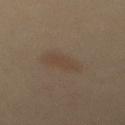biopsy status = total-body-photography surveillance lesion; no biopsy | automated metrics = a lesion area of about 6.5 mm² and a shape eccentricity near 0.9; a lesion–skin lightness drop of about 5 and a normalized border contrast of about 5; a border-irregularity index near 3/10, a within-lesion color-variation index near 1.5/10, and radial color variation of about 0.5 | illumination = cross-polarized illumination | anatomic site = the mid back | patient = female, aged 38–42 | diameter = about 4.5 mm | imaging modality = ~15 mm tile from a whole-body skin photo.